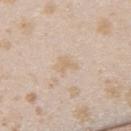automated lesion analysis: a border-irregularity index near 4.5/10 and radial color variation of about 0.5
subject: female, aged 23 to 27
diameter: ~2.5 mm (longest diameter)
site: the upper back
image: ~15 mm crop, total-body skin-cancer survey
lighting: white-light